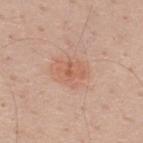Notes:
• notes: catalogued during a skin exam; not biopsied
• size: about 3 mm
• subject: male, about 40 years old
• TBP lesion metrics: a mean CIELAB color near L≈60 a*≈23 b*≈31, a lesion–skin lightness drop of about 7, and a normalized border contrast of about 5.5
• lighting: white-light
• body site: the upper back
• imaging modality: ~15 mm crop, total-body skin-cancer survey